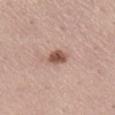Assessment:
The lesion was tiled from a total-body skin photograph and was not biopsied.
Context:
A female patient about 45 years old. A lesion tile, about 15 mm wide, cut from a 3D total-body photograph. From the right lower leg.